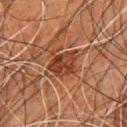Captured during whole-body skin photography for melanoma surveillance; the lesion was not biopsied. The lesion is located on the chest. The tile uses cross-polarized illumination. The recorded lesion diameter is about 3 mm. A 15 mm close-up extracted from a 3D total-body photography capture. An algorithmic analysis of the crop reported an area of roughly 8.5 mm², a shape eccentricity near 0.4, and a shape-asymmetry score of about 0.3 (0 = symmetric). It also reported an automated nevus-likeness rating near 35 out of 100. The subject is a male aged 78 to 82.